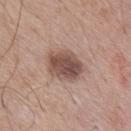Impression:
Recorded during total-body skin imaging; not selected for excision or biopsy.
Background:
A close-up tile cropped from a whole-body skin photograph, about 15 mm across. The subject is a male aged around 70. About 4.5 mm across. The total-body-photography lesion software estimated an outline eccentricity of about 0.65 (0 = round, 1 = elongated) and two-axis asymmetry of about 0.15. The software also gave a normalized border contrast of about 9.5. The lesion is on the upper back.